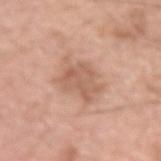<record>
  <biopsy_status>not biopsied; imaged during a skin examination</biopsy_status>
  <site>left upper arm</site>
  <image>
    <source>total-body photography crop</source>
    <field_of_view_mm>15</field_of_view_mm>
  </image>
  <patient>
    <sex>male</sex>
    <age_approx>20</age_approx>
  </patient>
</record>